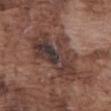Q: Automated lesion metrics?
A: an outline eccentricity of about 0.85 (0 = round, 1 = elongated) and two-axis asymmetry of about 0.4; a mean CIELAB color near L≈37 a*≈17 b*≈20, a lesion–skin lightness drop of about 10, and a normalized border contrast of about 9; internal color variation of about 8.5 on a 0–10 scale and a peripheral color-asymmetry measure near 2.5; a nevus-likeness score of about 0/100
Q: How was the tile lit?
A: white-light
Q: What is the imaging modality?
A: ~15 mm crop, total-body skin-cancer survey
Q: Who is the patient?
A: male, in their mid-70s
Q: What is the anatomic site?
A: the front of the torso